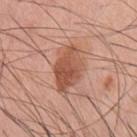workup — imaged on a skin check; not biopsied
TBP lesion metrics — a mean CIELAB color near L≈53 a*≈25 b*≈31 and a lesion-to-skin contrast of about 8 (normalized; higher = more distinct); a border-irregularity rating of about 2.5/10 and a peripheral color-asymmetry measure near 2; a lesion-detection confidence of about 100/100
diameter — ~5.5 mm (longest diameter)
tile lighting — white-light
anatomic site — the chest
acquisition — total-body-photography crop, ~15 mm field of view
patient — male, roughly 60 years of age